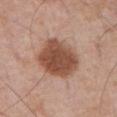{"biopsy_status": "not biopsied; imaged during a skin examination", "patient": {"sex": "male", "age_approx": 70}, "image": {"source": "total-body photography crop", "field_of_view_mm": 15}, "lighting": "white-light", "automated_metrics": {"peripheral_color_asymmetry": 1.0}, "site": "abdomen", "lesion_size": {"long_diameter_mm_approx": 5.5}}